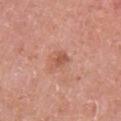follow-up: catalogued during a skin exam; not biopsied | patient: female, roughly 50 years of age | anatomic site: the right upper arm | tile lighting: white-light | imaging modality: ~15 mm tile from a whole-body skin photo | automated lesion analysis: a mean CIELAB color near L≈56 a*≈26 b*≈31, roughly 9 lightness units darker than nearby skin, and a normalized lesion–skin contrast near 6; an automated nevus-likeness rating near 0 out of 100 and a detector confidence of about 100 out of 100 that the crop contains a lesion.